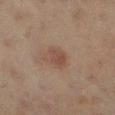workup: no biopsy performed (imaged during a skin exam)
size: about 2.5 mm
illumination: cross-polarized illumination
automated metrics: a lesion color around L≈40 a*≈17 b*≈24 in CIELAB, a lesion–skin lightness drop of about 6, and a lesion-to-skin contrast of about 6 (normalized; higher = more distinct); a border-irregularity index near 2/10, internal color variation of about 2.5 on a 0–10 scale, and a peripheral color-asymmetry measure near 1
acquisition: 15 mm crop, total-body photography
patient: female, in their mid-50s
anatomic site: the left lower leg